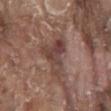Imaged during a routine full-body skin examination; the lesion was not biopsied and no histopathology is available.
The total-body-photography lesion software estimated a lesion color around L≈42 a*≈19 b*≈22 in CIELAB and a lesion-to-skin contrast of about 8 (normalized; higher = more distinct).
Captured under white-light illumination.
A 15 mm close-up tile from a total-body photography series done for melanoma screening.
From the front of the torso.
A male subject about 80 years old.